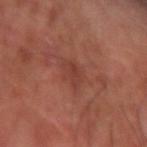Captured during whole-body skin photography for melanoma surveillance; the lesion was not biopsied. The lesion's longest dimension is about 3 mm. Captured under cross-polarized illumination. A 15 mm close-up tile from a total-body photography series done for melanoma screening. A male subject aged 58–62. From the arm.